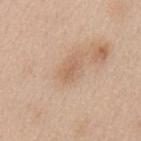<tbp_lesion>
  <image>
    <source>total-body photography crop</source>
    <field_of_view_mm>15</field_of_view_mm>
  </image>
  <lesion_size>
    <long_diameter_mm_approx>2.5</long_diameter_mm_approx>
  </lesion_size>
  <lighting>white-light</lighting>
  <automated_metrics>
    <area_mm2_approx>3.0</area_mm2_approx>
    <eccentricity>0.85</eccentricity>
    <shape_asymmetry>0.3</shape_asymmetry>
    <border_irregularity_0_10>3.0</border_irregularity_0_10>
    <color_variation_0_10>0.5</color_variation_0_10>
  </automated_metrics>
  <patient>
    <sex>female</sex>
    <age_approx>40</age_approx>
  </patient>
  <site>mid back</site>
</tbp_lesion>